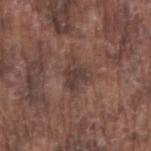biopsy status = no biopsy performed (imaged during a skin exam); site = the right upper arm; diameter = ≈3.5 mm; subject = male, approximately 75 years of age; imaging modality = 15 mm crop, total-body photography; lighting = white-light illumination.